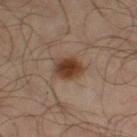| key | value |
|---|---|
| notes | no biopsy performed (imaged during a skin exam) |
| lighting | cross-polarized illumination |
| TBP lesion metrics | an area of roughly 8 mm² and two-axis asymmetry of about 0.15; a border-irregularity rating of about 1.5/10, a color-variation rating of about 4.5/10, and radial color variation of about 1.5 |
| lesion diameter | about 3.5 mm |
| location | the leg |
| subject | male, aged around 65 |
| image source | ~15 mm crop, total-body skin-cancer survey |